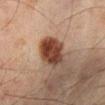Recorded during total-body skin imaging; not selected for excision or biopsy.
A 15 mm close-up extracted from a 3D total-body photography capture.
A male subject, in their mid-40s.
From the left lower leg.